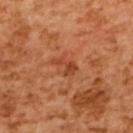No biopsy was performed on this lesion — it was imaged during a full skin examination and was not determined to be concerning.